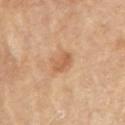Case summary:
– workup: total-body-photography surveillance lesion; no biopsy
– anatomic site: the right upper arm
– illumination: cross-polarized
– acquisition: total-body-photography crop, ~15 mm field of view
– subject: male, aged around 70